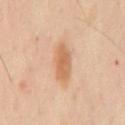Assessment: The lesion was photographed on a routine skin check and not biopsied; there is no pathology result.